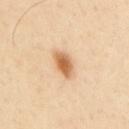– workup — no biopsy performed (imaged during a skin exam)
– lesion size — ≈3.5 mm
– patient — male, roughly 30 years of age
– lighting — cross-polarized illumination
– image source — 15 mm crop, total-body photography
– image-analysis metrics — an area of roughly 6 mm², a shape eccentricity near 0.85, and a shape-asymmetry score of about 0.2 (0 = symmetric); a mean CIELAB color near L≈65 a*≈21 b*≈40, roughly 15 lightness units darker than nearby skin, and a normalized border contrast of about 9; a border-irregularity index near 2/10, internal color variation of about 4 on a 0–10 scale, and peripheral color asymmetry of about 1
– site — the upper back